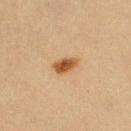Findings:
* notes — catalogued during a skin exam; not biopsied
* subject — female, aged around 40
* lighting — cross-polarized illumination
* imaging modality — ~15 mm tile from a whole-body skin photo
* location — the right lower leg
* diameter — ~3 mm (longest diameter)